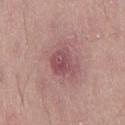A male patient aged approximately 45. The lesion is located on the right thigh. A 15 mm close-up tile from a total-body photography series done for melanoma screening. The lesion's longest dimension is about 3.5 mm.